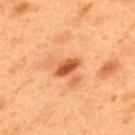notes: total-body-photography surveillance lesion; no biopsy
acquisition: ~15 mm tile from a whole-body skin photo
subject: male, aged approximately 50
body site: the back